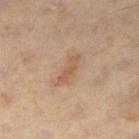Q: Was this lesion biopsied?
A: imaged on a skin check; not biopsied
Q: What is the anatomic site?
A: the left thigh
Q: Illumination type?
A: cross-polarized
Q: How large is the lesion?
A: ~3.5 mm (longest diameter)
Q: Who is the patient?
A: male, aged around 60
Q: What is the imaging modality?
A: ~15 mm crop, total-body skin-cancer survey